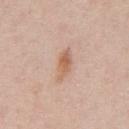Q: Is there a histopathology result?
A: no biopsy performed (imaged during a skin exam)
Q: What lighting was used for the tile?
A: white-light illumination
Q: How large is the lesion?
A: about 4 mm
Q: Who is the patient?
A: male, aged around 60
Q: Automated lesion metrics?
A: a lesion area of about 5.5 mm², an eccentricity of roughly 0.9, and a symmetry-axis asymmetry near 0.2; an average lesion color of about L≈62 a*≈20 b*≈31 (CIELAB), roughly 10 lightness units darker than nearby skin, and a normalized lesion–skin contrast near 7; border irregularity of about 2.5 on a 0–10 scale and a peripheral color-asymmetry measure near 1
Q: Lesion location?
A: the abdomen
Q: What kind of image is this?
A: ~15 mm tile from a whole-body skin photo